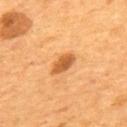Notes:
- follow-up · catalogued during a skin exam; not biopsied
- lesion diameter · ≈3.5 mm
- patient · male, aged around 55
- site · the upper back
- acquisition · ~15 mm tile from a whole-body skin photo